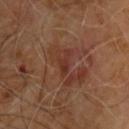Impression:
Part of a total-body skin-imaging series; this lesion was reviewed on a skin check and was not flagged for biopsy.
Background:
The patient is a male in their 60s. Captured under cross-polarized illumination. Approximately 3 mm at its widest. The total-body-photography lesion software estimated an area of roughly 3 mm² and an outline eccentricity of about 0.85 (0 = round, 1 = elongated). The software also gave border irregularity of about 5 on a 0–10 scale, a within-lesion color-variation index near 0.5/10, and peripheral color asymmetry of about 0. The software also gave a nevus-likeness score of about 0/100 and a detector confidence of about 100 out of 100 that the crop contains a lesion. A roughly 15 mm field-of-view crop from a total-body skin photograph. The lesion is located on the upper back.